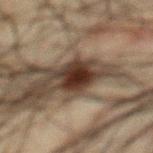The lesion was tiled from a total-body skin photograph and was not biopsied. This is a cross-polarized tile. The subject is a male roughly 60 years of age. The lesion is located on the chest. Cropped from a total-body skin-imaging series; the visible field is about 15 mm. The recorded lesion diameter is about 4 mm.